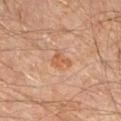notes: no biopsy performed (imaged during a skin exam) | size: ~2.5 mm (longest diameter) | image source: ~15 mm tile from a whole-body skin photo | anatomic site: the right forearm | illumination: cross-polarized | subject: male, approximately 45 years of age.